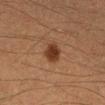Assessment:
Part of a total-body skin-imaging series; this lesion was reviewed on a skin check and was not flagged for biopsy.
Background:
Located on the right lower leg. The subject is a male roughly 40 years of age. The tile uses cross-polarized illumination. A roughly 15 mm field-of-view crop from a total-body skin photograph. About 2.5 mm across.